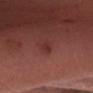Assessment: Part of a total-body skin-imaging series; this lesion was reviewed on a skin check and was not flagged for biopsy. Clinical summary: On the head or neck. The recorded lesion diameter is about 1.5 mm. The patient is a male aged 38–42. The total-body-photography lesion software estimated an outline eccentricity of about 0.7 (0 = round, 1 = elongated) and two-axis asymmetry of about 0.35. And it measured an average lesion color of about L≈32 a*≈26 b*≈25 (CIELAB), a lesion–skin lightness drop of about 6, and a lesion-to-skin contrast of about 6 (normalized; higher = more distinct). The software also gave a border-irregularity index near 3/10. A 15 mm close-up tile from a total-body photography series done for melanoma screening.